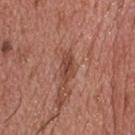No biopsy was performed on this lesion — it was imaged during a full skin examination and was not determined to be concerning. A male subject about 55 years old. Imaged with white-light lighting. The lesion is located on the head or neck. A roughly 15 mm field-of-view crop from a total-body skin photograph. An algorithmic analysis of the crop reported a nevus-likeness score of about 75/100 and a lesion-detection confidence of about 100/100.